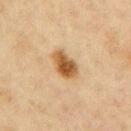Impression:
No biopsy was performed on this lesion — it was imaged during a full skin examination and was not determined to be concerning.
Clinical summary:
Measured at roughly 3.5 mm in maximum diameter. Located on the right upper arm. A female patient, in their mid-40s. Imaged with cross-polarized lighting. A 15 mm close-up extracted from a 3D total-body photography capture.